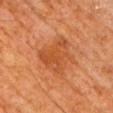{
  "biopsy_status": "not biopsied; imaged during a skin examination",
  "image": {
    "source": "total-body photography crop",
    "field_of_view_mm": 15
  },
  "site": "arm",
  "lighting": "cross-polarized",
  "patient": {
    "sex": "male",
    "age_approx": 65
  }
}